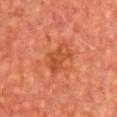The lesion was photographed on a routine skin check and not biopsied; there is no pathology result.
About 3.5 mm across.
A male patient, aged 63 to 67.
The total-body-photography lesion software estimated an eccentricity of roughly 0.75 and two-axis asymmetry of about 0.3.
A roughly 15 mm field-of-view crop from a total-body skin photograph.
The tile uses cross-polarized illumination.
On the chest.